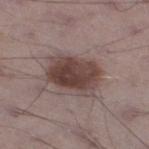{"biopsy_status": "not biopsied; imaged during a skin examination", "automated_metrics": {"cielab_L": 42, "cielab_a": 16, "cielab_b": 19, "vs_skin_darker_L": 13.0, "vs_skin_contrast_norm": 10.0, "border_irregularity_0_10": 1.5, "color_variation_0_10": 5.5, "peripheral_color_asymmetry": 2.0, "nevus_likeness_0_100": 90, "lesion_detection_confidence_0_100": 100}, "image": {"source": "total-body photography crop", "field_of_view_mm": 15}, "patient": {"sex": "male", "age_approx": 70}, "site": "left lower leg", "lesion_size": {"long_diameter_mm_approx": 5.5}}